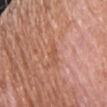Clinical impression:
Part of a total-body skin-imaging series; this lesion was reviewed on a skin check and was not flagged for biopsy.
Context:
A 15 mm crop from a total-body photograph taken for skin-cancer surveillance. Automated tile analysis of the lesion measured a shape eccentricity near 0.9 and a shape-asymmetry score of about 0.4 (0 = symmetric). The software also gave a lesion color around L≈55 a*≈25 b*≈33 in CIELAB and roughly 6 lightness units darker than nearby skin. And it measured border irregularity of about 5 on a 0–10 scale and internal color variation of about 0 on a 0–10 scale. It also reported a classifier nevus-likeness of about 0/100 and a lesion-detection confidence of about 95/100. From the chest. A male subject aged approximately 65. Imaged with white-light lighting. The lesion's longest dimension is about 2.5 mm.